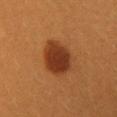Impression: This lesion was catalogued during total-body skin photography and was not selected for biopsy. Context: A female patient aged approximately 30. A lesion tile, about 15 mm wide, cut from a 3D total-body photograph. From the chest. The recorded lesion diameter is about 5 mm. This is a cross-polarized tile.